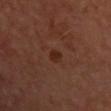Recorded during total-body skin imaging; not selected for excision or biopsy.
A male subject, in their mid- to late 60s.
A 15 mm close-up tile from a total-body photography series done for melanoma screening.
The lesion is located on the chest.